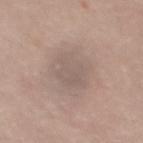Findings:
• workup · imaged on a skin check; not biopsied
• automated lesion analysis · an average lesion color of about L≈57 a*≈13 b*≈22 (CIELAB), roughly 6 lightness units darker than nearby skin, and a normalized border contrast of about 4.5; border irregularity of about 2.5 on a 0–10 scale, a color-variation rating of about 2/10, and peripheral color asymmetry of about 0.5
• image source · ~15 mm crop, total-body skin-cancer survey
• body site · the upper back
• patient · female, aged 38–42
• lesion diameter · about 4.5 mm
• illumination · white-light illumination